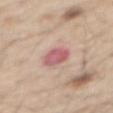Assessment: Captured during whole-body skin photography for melanoma surveillance; the lesion was not biopsied. Background: The patient is a male aged 68–72. The lesion is located on the abdomen. Captured under white-light illumination. Automated image analysis of the tile measured a footprint of about 6.5 mm², a shape eccentricity near 0.65, and two-axis asymmetry of about 0.2. The analysis additionally found a normalized lesion–skin contrast near 8.5. The analysis additionally found a classifier nevus-likeness of about 5/100. Measured at roughly 3 mm in maximum diameter. A 15 mm crop from a total-body photograph taken for skin-cancer surveillance.